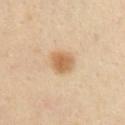Impression:
Captured during whole-body skin photography for melanoma surveillance; the lesion was not biopsied.
Context:
Cropped from a whole-body photographic skin survey; the tile spans about 15 mm. The lesion is located on the right upper arm. The lesion-visualizer software estimated an area of roughly 7 mm², a shape eccentricity near 0.65, and two-axis asymmetry of about 0.2. It also reported a mean CIELAB color near L≈62 a*≈17 b*≈37, roughly 11 lightness units darker than nearby skin, and a lesion-to-skin contrast of about 8 (normalized; higher = more distinct). A male patient, about 45 years old. This is a cross-polarized tile. The recorded lesion diameter is about 3.5 mm.